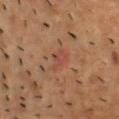Captured during whole-body skin photography for melanoma surveillance; the lesion was not biopsied.
On the chest.
The tile uses cross-polarized illumination.
The lesion's longest dimension is about 3 mm.
A 15 mm crop from a total-body photograph taken for skin-cancer surveillance.
The subject is a male aged 53–57.